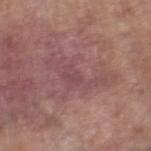<tbp_lesion>
  <biopsy_status>not biopsied; imaged during a skin examination</biopsy_status>
  <site>right thigh</site>
  <patient>
    <sex>male</sex>
    <age_approx>80</age_approx>
  </patient>
  <image>
    <source>total-body photography crop</source>
    <field_of_view_mm>15</field_of_view_mm>
  </image>
  <lesion_size>
    <long_diameter_mm_approx>7.5</long_diameter_mm_approx>
  </lesion_size>
  <automated_metrics>
    <eccentricity>0.9</eccentricity>
    <shape_asymmetry>0.65</shape_asymmetry>
    <border_irregularity_0_10>9.5</border_irregularity_0_10>
    <color_variation_0_10>2.0</color_variation_0_10>
    <peripheral_color_asymmetry>0.5</peripheral_color_asymmetry>
  </automated_metrics>
  <lighting>white-light</lighting>
</tbp_lesion>